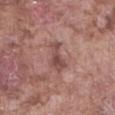Findings:
* notes — total-body-photography surveillance lesion; no biopsy
* patient — male, about 75 years old
* site — the front of the torso
* illumination — white-light illumination
* lesion size — ≈4 mm
* automated lesion analysis — a lesion color around L≈47 a*≈22 b*≈22 in CIELAB, about 10 CIELAB-L* units darker than the surrounding skin, and a lesion-to-skin contrast of about 7.5 (normalized; higher = more distinct); a border-irregularity index near 5.5/10, a within-lesion color-variation index near 2.5/10, and peripheral color asymmetry of about 0.5
* image source — ~15 mm crop, total-body skin-cancer survey